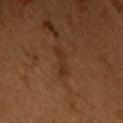Recorded during total-body skin imaging; not selected for excision or biopsy. The tile uses cross-polarized illumination. This image is a 15 mm lesion crop taken from a total-body photograph. The lesion is located on the chest. Longest diameter approximately 3.5 mm. A male patient, aged 48–52.